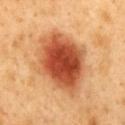{
  "biopsy_status": "not biopsied; imaged during a skin examination",
  "lesion_size": {
    "long_diameter_mm_approx": 7.0
  },
  "patient": {
    "sex": "male",
    "age_approx": 50
  },
  "automated_metrics": {
    "eccentricity": 0.65,
    "shape_asymmetry": 0.1,
    "cielab_L": 52,
    "cielab_a": 31,
    "cielab_b": 40,
    "vs_skin_darker_L": 18.0,
    "vs_skin_contrast_norm": 11.5,
    "nevus_likeness_0_100": 100,
    "lesion_detection_confidence_0_100": 100
  },
  "lighting": "cross-polarized",
  "site": "mid back",
  "image": {
    "source": "total-body photography crop",
    "field_of_view_mm": 15
  }
}